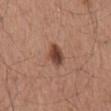| feature | finding |
|---|---|
| follow-up | catalogued during a skin exam; not biopsied |
| diameter | ≈3 mm |
| patient | male, approximately 75 years of age |
| image | ~15 mm tile from a whole-body skin photo |
| TBP lesion metrics | an eccentricity of roughly 0.55; a border-irregularity rating of about 2/10 and a peripheral color-asymmetry measure near 1.5; a classifier nevus-likeness of about 95/100 and lesion-presence confidence of about 100/100 |
| location | the mid back |